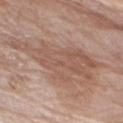Notes:
* notes — catalogued during a skin exam; not biopsied
* diameter — about 12.5 mm
* site — the right upper arm
* lighting — white-light
* subject — male, roughly 80 years of age
* acquisition — 15 mm crop, total-body photography